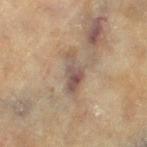  biopsy_status: not biopsied; imaged during a skin examination
  automated_metrics:
    cielab_L: 44
    cielab_a: 13
    cielab_b: 21
    vs_skin_darker_L: 9.0
    vs_skin_contrast_norm: 8.0
    border_irregularity_0_10: 4.5
    color_variation_0_10: 4.0
    peripheral_color_asymmetry: 1.0
  lighting: cross-polarized
  site: left thigh
  patient:
    sex: female
    age_approx: 80
  lesion_size:
    long_diameter_mm_approx: 4.5
  image:
    source: total-body photography crop
    field_of_view_mm: 15